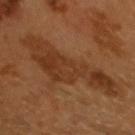  biopsy_status: not biopsied; imaged during a skin examination
  patient:
    sex: male
    age_approx: 60
  image:
    source: total-body photography crop
    field_of_view_mm: 15
  site: head or neck